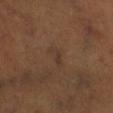<record>
<biopsy_status>not biopsied; imaged during a skin examination</biopsy_status>
<image>
  <source>total-body photography crop</source>
  <field_of_view_mm>15</field_of_view_mm>
</image>
<site>leg</site>
<patient>
  <sex>male</sex>
  <age_approx>50</age_approx>
</patient>
<lighting>cross-polarized</lighting>
</record>